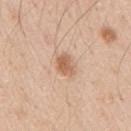Clinical impression: This lesion was catalogued during total-body skin photography and was not selected for biopsy. Image and clinical context: This image is a 15 mm lesion crop taken from a total-body photograph. The lesion is located on the arm. A male patient approximately 35 years of age.